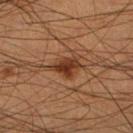workup: catalogued during a skin exam; not biopsied | image-analysis metrics: an eccentricity of roughly 0.7 and two-axis asymmetry of about 0.25 | image source: 15 mm crop, total-body photography | anatomic site: the right lower leg | tile lighting: cross-polarized illumination | subject: male, approximately 45 years of age | size: about 3.5 mm.